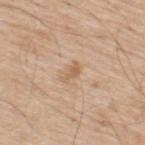Captured during whole-body skin photography for melanoma surveillance; the lesion was not biopsied.
A male patient aged approximately 70.
On the upper back.
A lesion tile, about 15 mm wide, cut from a 3D total-body photograph.